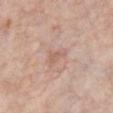Q: What are the patient's age and sex?
A: male, aged 58 to 62
Q: How large is the lesion?
A: about 3 mm
Q: What kind of image is this?
A: 15 mm crop, total-body photography
Q: Where on the body is the lesion?
A: the front of the torso
Q: How was the tile lit?
A: white-light illumination